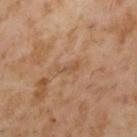follow-up = catalogued during a skin exam; not biopsied | tile lighting = cross-polarized illumination | location = the left upper arm | acquisition = 15 mm crop, total-body photography | patient = male, in their mid-50s | automated metrics = a footprint of about 2 mm²; a mean CIELAB color near L≈52 a*≈20 b*≈33 and about 7 CIELAB-L* units darker than the surrounding skin.